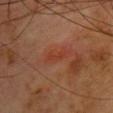biopsy status — catalogued during a skin exam; not biopsied | body site — the front of the torso | imaging modality — 15 mm crop, total-body photography | illumination — cross-polarized | lesion diameter — ~3 mm (longest diameter) | patient — female, aged 53–57 | image-analysis metrics — an area of roughly 3.5 mm², a shape eccentricity near 0.95, and a symmetry-axis asymmetry near 0.4; a nevus-likeness score of about 0/100 and a detector confidence of about 100 out of 100 that the crop contains a lesion.